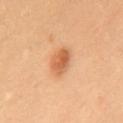Imaged during a routine full-body skin examination; the lesion was not biopsied and no histopathology is available. The patient is a male in their mid-60s. A region of skin cropped from a whole-body photographic capture, roughly 15 mm wide. An algorithmic analysis of the crop reported a lesion color around L≈62 a*≈26 b*≈40 in CIELAB, a lesion–skin lightness drop of about 12, and a normalized lesion–skin contrast near 7.5. And it measured a detector confidence of about 100 out of 100 that the crop contains a lesion. Longest diameter approximately 3.5 mm. On the chest.